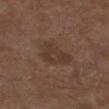Impression: Imaged during a routine full-body skin examination; the lesion was not biopsied and no histopathology is available. Context: A 15 mm crop from a total-body photograph taken for skin-cancer surveillance. Approximately 4.5 mm at its widest. Located on the left lower leg. The total-body-photography lesion software estimated a footprint of about 11 mm², an outline eccentricity of about 0.7 (0 = round, 1 = elongated), and a symmetry-axis asymmetry near 0.35. It also reported an average lesion color of about L≈36 a*≈16 b*≈23 (CIELAB) and roughly 6 lightness units darker than nearby skin. It also reported a classifier nevus-likeness of about 0/100 and lesion-presence confidence of about 100/100. This is a white-light tile. The subject is a male aged 73–77.